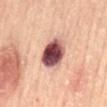Clinical impression: This lesion was catalogued during total-body skin photography and was not selected for biopsy. Clinical summary: From the abdomen. An algorithmic analysis of the crop reported a mean CIELAB color near L≈51 a*≈25 b*≈21, about 24 CIELAB-L* units darker than the surrounding skin, and a lesion-to-skin contrast of about 15.5 (normalized; higher = more distinct). The analysis additionally found an automated nevus-likeness rating near 95 out of 100 and lesion-presence confidence of about 100/100. Longest diameter approximately 4.5 mm. A 15 mm crop from a total-body photograph taken for skin-cancer surveillance. A male patient aged approximately 65. Captured under cross-polarized illumination.